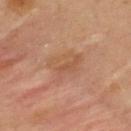biopsy status=imaged on a skin check; not biopsied | TBP lesion metrics=an eccentricity of roughly 0.65 and two-axis asymmetry of about 0.3; a border-irregularity index near 3.5/10, a within-lesion color-variation index near 4/10, and a peripheral color-asymmetry measure near 1.5 | acquisition=~15 mm crop, total-body skin-cancer survey | patient=female, aged 33 to 37 | anatomic site=the upper back.